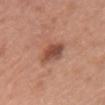notes: imaged on a skin check; not biopsied
image-analysis metrics: an outline eccentricity of about 0.75 (0 = round, 1 = elongated) and a shape-asymmetry score of about 0.2 (0 = symmetric); a lesion color around L≈49 a*≈25 b*≈29 in CIELAB and a normalized border contrast of about 8.5; border irregularity of about 2 on a 0–10 scale, a color-variation rating of about 4/10, and peripheral color asymmetry of about 1; an automated nevus-likeness rating near 90 out of 100 and a lesion-detection confidence of about 100/100
anatomic site: the chest
diameter: ~3.5 mm (longest diameter)
imaging modality: total-body-photography crop, ~15 mm field of view
patient: female, roughly 60 years of age
tile lighting: white-light illumination